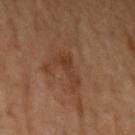Imaged during a routine full-body skin examination; the lesion was not biopsied and no histopathology is available. The patient is a female aged 63–67. A roughly 15 mm field-of-view crop from a total-body skin photograph. The lesion is on the left upper arm.